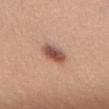Q: Was a biopsy performed?
A: total-body-photography surveillance lesion; no biopsy
Q: What is the anatomic site?
A: the lower back
Q: Patient demographics?
A: female, approximately 25 years of age
Q: How was the tile lit?
A: white-light illumination
Q: How was this image acquired?
A: 15 mm crop, total-body photography
Q: Lesion size?
A: ~3.5 mm (longest diameter)